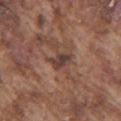patient = male, in their mid- to late 70s; site = the arm; image source = ~15 mm tile from a whole-body skin photo.